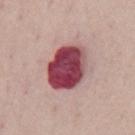notes — total-body-photography surveillance lesion; no biopsy
illumination — white-light
anatomic site — the chest
size — ≈6 mm
automated lesion analysis — an eccentricity of roughly 0.7 and a shape-asymmetry score of about 0.15 (0 = symmetric); an average lesion color of about L≈45 a*≈32 b*≈17 (CIELAB), a lesion–skin lightness drop of about 23, and a normalized border contrast of about 15.5; a nevus-likeness score of about 0/100 and a lesion-detection confidence of about 100/100
acquisition — total-body-photography crop, ~15 mm field of view
subject — male, approximately 50 years of age